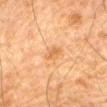This lesion was catalogued during total-body skin photography and was not selected for biopsy. A male patient, aged around 80. Longest diameter approximately 2.5 mm. The tile uses cross-polarized illumination. Cropped from a total-body skin-imaging series; the visible field is about 15 mm. The lesion is on the abdomen.